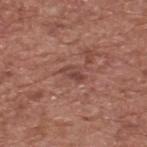notes: total-body-photography surveillance lesion; no biopsy
body site: the upper back
patient: male, in their mid-70s
acquisition: total-body-photography crop, ~15 mm field of view